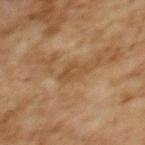Part of a total-body skin-imaging series; this lesion was reviewed on a skin check and was not flagged for biopsy.
This image is a 15 mm lesion crop taken from a total-body photograph.
Automated image analysis of the tile measured a footprint of about 4 mm², a shape eccentricity near 0.9, and a shape-asymmetry score of about 0.45 (0 = symmetric). And it measured a border-irregularity index near 5/10, a within-lesion color-variation index near 1.5/10, and radial color variation of about 0.5.
This is a cross-polarized tile.
A female subject, aged 58 to 62.
Measured at roughly 3.5 mm in maximum diameter.
The lesion is located on the upper back.